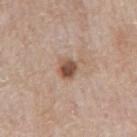subject = male, in their mid- to late 60s
imaging modality = ~15 mm tile from a whole-body skin photo
diameter = ~3 mm (longest diameter)
anatomic site = the mid back
automated lesion analysis = an eccentricity of roughly 0.65 and a symmetry-axis asymmetry near 0.2